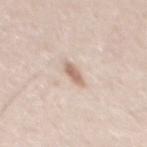Imaged during a routine full-body skin examination; the lesion was not biopsied and no histopathology is available. On the mid back. Captured under white-light illumination. The subject is a male approximately 35 years of age. A 15 mm crop from a total-body photograph taken for skin-cancer surveillance. The lesion's longest dimension is about 3 mm.